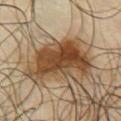Assessment:
The lesion was photographed on a routine skin check and not biopsied; there is no pathology result.
Clinical summary:
A region of skin cropped from a whole-body photographic capture, roughly 15 mm wide. The recorded lesion diameter is about 7.5 mm. Located on the chest. This is a cross-polarized tile. A male patient about 65 years old.